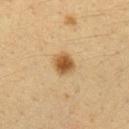Clinical impression: Imaged during a routine full-body skin examination; the lesion was not biopsied and no histopathology is available. Image and clinical context: The lesion is on the left forearm. A female patient roughly 30 years of age. Automated image analysis of the tile measured a shape eccentricity near 0.4 and two-axis asymmetry of about 0.15. The software also gave a lesion color around L≈50 a*≈18 b*≈38 in CIELAB, about 13 CIELAB-L* units darker than the surrounding skin, and a normalized lesion–skin contrast near 10. A 15 mm close-up tile from a total-body photography series done for melanoma screening. The lesion's longest dimension is about 2.5 mm.